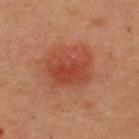The lesion was tiled from a total-body skin photograph and was not biopsied. The lesion is located on the back. Cropped from a whole-body photographic skin survey; the tile spans about 15 mm. A female subject in their mid-40s.